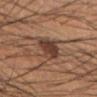Imaged during a routine full-body skin examination; the lesion was not biopsied and no histopathology is available.
Automated tile analysis of the lesion measured an eccentricity of roughly 0.55 and a shape-asymmetry score of about 0.3 (0 = symmetric). The analysis additionally found about 11 CIELAB-L* units darker than the surrounding skin.
The lesion is on the left lower leg.
A lesion tile, about 15 mm wide, cut from a 3D total-body photograph.
Captured under cross-polarized illumination.
A male subject in their mid- to late 40s.
Longest diameter approximately 4 mm.